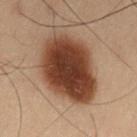<record>
  <biopsy_status>not biopsied; imaged during a skin examination</biopsy_status>
  <lesion_size>
    <long_diameter_mm_approx>7.5</long_diameter_mm_approx>
  </lesion_size>
  <patient>
    <sex>male</sex>
    <age_approx>55</age_approx>
  </patient>
  <site>left thigh</site>
  <image>
    <source>total-body photography crop</source>
    <field_of_view_mm>15</field_of_view_mm>
  </image>
  <automated_metrics>
    <cielab_L>32</cielab_L>
    <cielab_a>18</cielab_a>
    <cielab_b>25</cielab_b>
    <vs_skin_contrast_norm>14.0</vs_skin_contrast_norm>
    <nevus_likeness_0_100>100</nevus_likeness_0_100>
  </automated_metrics>
</record>